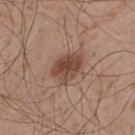| key | value |
|---|---|
| notes | imaged on a skin check; not biopsied |
| imaging modality | ~15 mm tile from a whole-body skin photo |
| illumination | white-light |
| location | the upper back |
| size | ≈4.5 mm |
| patient | male, aged 48 to 52 |